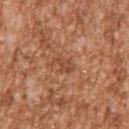No biopsy was performed on this lesion — it was imaged during a full skin examination and was not determined to be concerning. This image is a 15 mm lesion crop taken from a total-body photograph. From the right upper arm. The lesion-visualizer software estimated a lesion color around L≈48 a*≈25 b*≈33 in CIELAB, a lesion–skin lightness drop of about 8, and a normalized border contrast of about 6. The software also gave a border-irregularity rating of about 3.5/10, a color-variation rating of about 1.5/10, and radial color variation of about 0.5. Captured under white-light illumination. A male patient roughly 45 years of age.